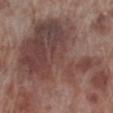• notes · imaged on a skin check; not biopsied
• image · ~15 mm crop, total-body skin-cancer survey
• location · the leg
• automated metrics · an average lesion color of about L≈43 a*≈19 b*≈21 (CIELAB) and a lesion–skin lightness drop of about 10
• patient · male, about 70 years old